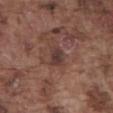Case summary:
- imaging modality · total-body-photography crop, ~15 mm field of view
- lighting · white-light illumination
- lesion size · ~2.5 mm (longest diameter)
- anatomic site · the abdomen
- patient · male, approximately 75 years of age
- automated metrics · a lesion area of about 4 mm², a shape eccentricity near 0.7, and a symmetry-axis asymmetry near 0.2; a nevus-likeness score of about 0/100